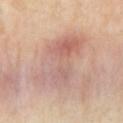Imaged during a routine full-body skin examination; the lesion was not biopsied and no histopathology is available.
A female patient aged approximately 40.
Captured under cross-polarized illumination.
A 15 mm close-up extracted from a 3D total-body photography capture.
Approximately 7.5 mm at its widest.
The lesion is located on the left upper arm.
The lesion-visualizer software estimated an average lesion color of about L≈63 a*≈20 b*≈25 (CIELAB), about 8 CIELAB-L* units darker than the surrounding skin, and a normalized border contrast of about 5. The software also gave a border-irregularity rating of about 4/10 and a peripheral color-asymmetry measure near 2.5. And it measured a classifier nevus-likeness of about 0/100 and a lesion-detection confidence of about 100/100.